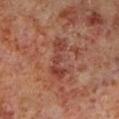notes = catalogued during a skin exam; not biopsied
lighting = cross-polarized
imaging modality = 15 mm crop, total-body photography
patient = male, aged around 60
anatomic site = the left lower leg
diameter = ~5 mm (longest diameter)
image-analysis metrics = a lesion area of about 8 mm² and a shape eccentricity near 0.95; a mean CIELAB color near L≈39 a*≈25 b*≈26 and about 8 CIELAB-L* units darker than the surrounding skin; a border-irregularity index near 6/10, internal color variation of about 3 on a 0–10 scale, and peripheral color asymmetry of about 1